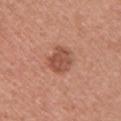{"biopsy_status": "not biopsied; imaged during a skin examination", "lesion_size": {"long_diameter_mm_approx": 3.5}, "automated_metrics": {"area_mm2_approx": 8.0, "eccentricity": 0.45, "nevus_likeness_0_100": 15, "lesion_detection_confidence_0_100": 100}, "lighting": "white-light", "site": "front of the torso", "image": {"source": "total-body photography crop", "field_of_view_mm": 15}, "patient": {"sex": "female", "age_approx": 40}}